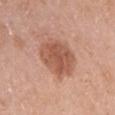{
  "image": {
    "source": "total-body photography crop",
    "field_of_view_mm": 15
  },
  "site": "left upper arm",
  "patient": {
    "sex": "female",
    "age_approx": 65
  },
  "lesion_size": {
    "long_diameter_mm_approx": 5.0
  }
}